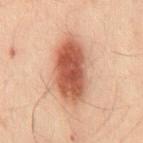  biopsy_status: not biopsied; imaged during a skin examination
  image:
    source: total-body photography crop
    field_of_view_mm: 15
  patient:
    sex: male
    age_approx: 50
  site: mid back